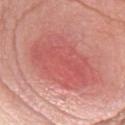| feature | finding |
|---|---|
| location | the chest |
| diameter | ~9 mm (longest diameter) |
| automated lesion analysis | border irregularity of about 2.5 on a 0–10 scale, a within-lesion color-variation index near 4/10, and radial color variation of about 1.5; an automated nevus-likeness rating near 85 out of 100 and lesion-presence confidence of about 100/100 |
| lighting | white-light |
| imaging modality | total-body-photography crop, ~15 mm field of view |
| subject | female, in their mid-50s |